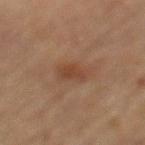{"biopsy_status": "not biopsied; imaged during a skin examination", "site": "left thigh", "lesion_size": {"long_diameter_mm_approx": 3.0}, "lighting": "cross-polarized", "automated_metrics": {"cielab_L": 37, "cielab_a": 18, "cielab_b": 27, "vs_skin_darker_L": 7.0, "vs_skin_contrast_norm": 6.5}, "image": {"source": "total-body photography crop", "field_of_view_mm": 15}, "patient": {"sex": "female", "age_approx": 55}}